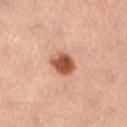{
  "biopsy_status": "not biopsied; imaged during a skin examination",
  "site": "leg",
  "patient": {
    "sex": "female",
    "age_approx": 35
  },
  "image": {
    "source": "total-body photography crop",
    "field_of_view_mm": 15
  }
}